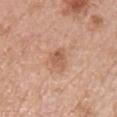Imaged during a routine full-body skin examination; the lesion was not biopsied and no histopathology is available.
A male patient aged approximately 65.
Located on the chest.
About 2.5 mm across.
This image is a 15 mm lesion crop taken from a total-body photograph.